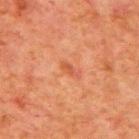biopsy_status: not biopsied; imaged during a skin examination
lighting: cross-polarized
image:
  source: total-body photography crop
  field_of_view_mm: 15
patient:
  sex: male
  age_approx: 80
site: mid back